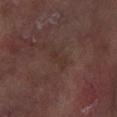* workup · imaged on a skin check; not biopsied
* lesion diameter · about 3 mm
* location · the arm
* image · 15 mm crop, total-body photography
* illumination · cross-polarized
* subject · female, approximately 80 years of age